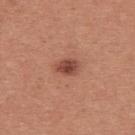Recorded during total-body skin imaging; not selected for excision or biopsy.
The lesion is on the upper back.
Cropped from a whole-body photographic skin survey; the tile spans about 15 mm.
A female patient, roughly 40 years of age.